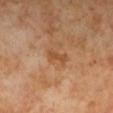Q: Was this lesion biopsied?
A: imaged on a skin check; not biopsied
Q: What did automated image analysis measure?
A: an area of roughly 3.5 mm² and an outline eccentricity of about 0.9 (0 = round, 1 = elongated); a mean CIELAB color near L≈51 a*≈23 b*≈38 and a normalized border contrast of about 6; a border-irregularity rating of about 3.5/10, internal color variation of about 1 on a 0–10 scale, and peripheral color asymmetry of about 0.5; a nevus-likeness score of about 0/100 and a lesion-detection confidence of about 100/100
Q: How was this image acquired?
A: total-body-photography crop, ~15 mm field of view
Q: Illumination type?
A: cross-polarized
Q: Where on the body is the lesion?
A: the left lower leg
Q: How large is the lesion?
A: about 3 mm
Q: Who is the patient?
A: female, approximately 50 years of age